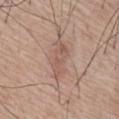No biopsy was performed on this lesion — it was imaged during a full skin examination and was not determined to be concerning. The lesion's longest dimension is about 5.5 mm. A roughly 15 mm field-of-view crop from a total-body skin photograph. The tile uses white-light illumination. A male subject in their mid- to late 70s. The lesion is on the front of the torso.